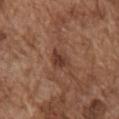Case summary:
• biopsy status: catalogued during a skin exam; not biopsied
• patient: male, aged 73–77
• lighting: white-light
• site: the right upper arm
• lesion size: ≈3 mm
• TBP lesion metrics: a lesion area of about 4.5 mm², a shape eccentricity near 0.6, and a symmetry-axis asymmetry near 0.3
• imaging modality: ~15 mm crop, total-body skin-cancer survey